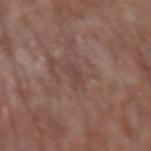This lesion was catalogued during total-body skin photography and was not selected for biopsy.
Cropped from a whole-body photographic skin survey; the tile spans about 15 mm.
Located on the left upper arm.
A male subject, about 65 years old.